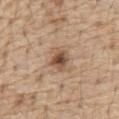Q: Was a biopsy performed?
A: no biopsy performed (imaged during a skin exam)
Q: Illumination type?
A: white-light
Q: Lesion size?
A: about 3 mm
Q: Who is the patient?
A: male, roughly 70 years of age
Q: What is the anatomic site?
A: the abdomen
Q: What kind of image is this?
A: 15 mm crop, total-body photography
Q: What did automated image analysis measure?
A: border irregularity of about 2.5 on a 0–10 scale, a color-variation rating of about 5.5/10, and peripheral color asymmetry of about 1.5